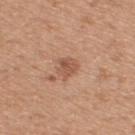Clinical impression: The lesion was photographed on a routine skin check and not biopsied; there is no pathology result. Context: A roughly 15 mm field-of-view crop from a total-body skin photograph. The patient is a male roughly 50 years of age. Located on the back.